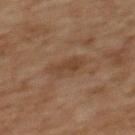Q: Is there a histopathology result?
A: catalogued during a skin exam; not biopsied
Q: Automated lesion metrics?
A: an area of roughly 6 mm² and two-axis asymmetry of about 0.2; a mean CIELAB color near L≈36 a*≈15 b*≈27, about 6 CIELAB-L* units darker than the surrounding skin, and a normalized lesion–skin contrast near 6; a detector confidence of about 100 out of 100 that the crop contains a lesion
Q: What lighting was used for the tile?
A: cross-polarized
Q: Who is the patient?
A: female, roughly 60 years of age
Q: How was this image acquired?
A: ~15 mm tile from a whole-body skin photo
Q: Lesion size?
A: ≈3.5 mm
Q: Lesion location?
A: the upper back